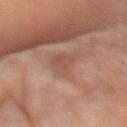Imaged during a routine full-body skin examination; the lesion was not biopsied and no histopathology is available.
From the left forearm.
Automated image analysis of the tile measured about 7 CIELAB-L* units darker than the surrounding skin. And it measured border irregularity of about 4 on a 0–10 scale, internal color variation of about 2.5 on a 0–10 scale, and a peripheral color-asymmetry measure near 1. The software also gave a classifier nevus-likeness of about 0/100 and lesion-presence confidence of about 100/100.
A female patient aged 68 to 72.
Approximately 3.5 mm at its widest.
A 15 mm crop from a total-body photograph taken for skin-cancer surveillance.
Captured under cross-polarized illumination.